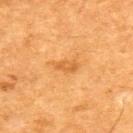| key | value |
|---|---|
| workup | total-body-photography surveillance lesion; no biopsy |
| patient | male, aged approximately 60 |
| anatomic site | the upper back |
| image source | ~15 mm tile from a whole-body skin photo |